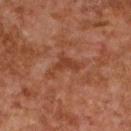Impression: Imaged during a routine full-body skin examination; the lesion was not biopsied and no histopathology is available. Clinical summary: From the upper back. Longest diameter approximately 4.5 mm. A female patient aged 58–62. A region of skin cropped from a whole-body photographic capture, roughly 15 mm wide. Imaged with cross-polarized lighting.